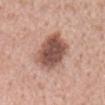This lesion was catalogued during total-body skin photography and was not selected for biopsy. The patient is a male approximately 60 years of age. Cropped from a total-body skin-imaging series; the visible field is about 15 mm. The lesion-visualizer software estimated a mean CIELAB color near L≈52 a*≈21 b*≈25 and about 16 CIELAB-L* units darker than the surrounding skin. The lesion's longest dimension is about 6 mm. Captured under white-light illumination. On the front of the torso.